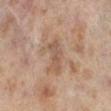Q: Was this lesion biopsied?
A: catalogued during a skin exam; not biopsied
Q: What is the imaging modality?
A: ~15 mm tile from a whole-body skin photo
Q: What are the patient's age and sex?
A: female, about 40 years old
Q: Lesion location?
A: the right lower leg